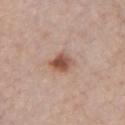biopsy_status: not biopsied; imaged during a skin examination
site: chest
lesion_size:
  long_diameter_mm_approx: 2.5
patient:
  sex: female
  age_approx: 65
lighting: white-light
image:
  source: total-body photography crop
  field_of_view_mm: 15